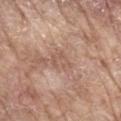This lesion was catalogued during total-body skin photography and was not selected for biopsy.
A male patient, in their mid- to late 60s.
An algorithmic analysis of the crop reported a lesion–skin lightness drop of about 7.
From the mid back.
A 15 mm crop from a total-body photograph taken for skin-cancer surveillance.
The lesion's longest dimension is about 2.5 mm.
This is a white-light tile.